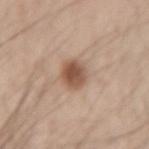Imaged during a routine full-body skin examination; the lesion was not biopsied and no histopathology is available.